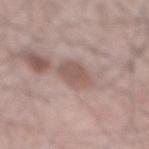| key | value |
|---|---|
| automated lesion analysis | a lesion area of about 7 mm², an outline eccentricity of about 0.8 (0 = round, 1 = elongated), and a symmetry-axis asymmetry near 0.2 |
| tile lighting | white-light illumination |
| site | the mid back |
| subject | male, roughly 65 years of age |
| image | 15 mm crop, total-body photography |